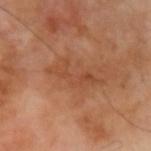No biopsy was performed on this lesion — it was imaged during a full skin examination and was not determined to be concerning. The tile uses cross-polarized illumination. Automated tile analysis of the lesion measured a footprint of about 8 mm² and a shape-asymmetry score of about 0.5 (0 = symmetric). It also reported an average lesion color of about L≈46 a*≈24 b*≈34 (CIELAB). Cropped from a whole-body photographic skin survey; the tile spans about 15 mm. The patient is a male approximately 70 years of age. The recorded lesion diameter is about 6 mm. Located on the left upper arm.